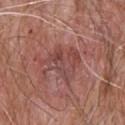{"biopsy_status": "not biopsied; imaged during a skin examination", "lesion_size": {"long_diameter_mm_approx": 5.0}, "automated_metrics": {"area_mm2_approx": 14.0, "eccentricity": 0.6, "shape_asymmetry": 0.4, "cielab_L": 46, "cielab_a": 24, "cielab_b": 23, "vs_skin_darker_L": 7.0, "vs_skin_contrast_norm": 5.5}, "image": {"source": "total-body photography crop", "field_of_view_mm": 15}, "site": "upper back", "lighting": "white-light", "patient": {"sex": "male", "age_approx": 80}}